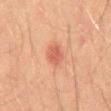notes: total-body-photography surveillance lesion; no biopsy | subject: male, aged approximately 45 | image-analysis metrics: a footprint of about 4 mm² and a symmetry-axis asymmetry near 0.3; an average lesion color of about L≈49 a*≈25 b*≈28 (CIELAB) and a lesion-to-skin contrast of about 6.5 (normalized; higher = more distinct); a border-irregularity rating of about 2.5/10, a color-variation rating of about 2.5/10, and radial color variation of about 1 | image source: total-body-photography crop, ~15 mm field of view | lighting: cross-polarized | body site: the mid back.